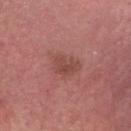Assessment: Recorded during total-body skin imaging; not selected for excision or biopsy. Clinical summary: The patient is a male in their 50s. The lesion is located on the head or neck. A 15 mm crop from a total-body photograph taken for skin-cancer surveillance. Longest diameter approximately 3.5 mm.